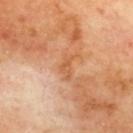Part of a total-body skin-imaging series; this lesion was reviewed on a skin check and was not flagged for biopsy. A male subject aged around 70. A roughly 15 mm field-of-view crop from a total-body skin photograph. The lesion-visualizer software estimated an average lesion color of about L≈57 a*≈25 b*≈39 (CIELAB) and roughly 7 lightness units darker than nearby skin. The analysis additionally found an automated nevus-likeness rating near 0 out of 100 and a lesion-detection confidence of about 100/100. The recorded lesion diameter is about 3 mm. Located on the back. The tile uses cross-polarized illumination.